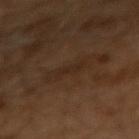{"biopsy_status": "not biopsied; imaged during a skin examination", "lesion_size": {"long_diameter_mm_approx": 2.5}, "image": {"source": "total-body photography crop", "field_of_view_mm": 15}, "automated_metrics": {"eccentricity": 0.9, "shape_asymmetry": 0.45, "cielab_L": 24, "cielab_a": 15, "cielab_b": 24, "vs_skin_darker_L": 4.0, "vs_skin_contrast_norm": 5.0, "border_irregularity_0_10": 5.0, "color_variation_0_10": 0.0, "peripheral_color_asymmetry": 0.0}, "patient": {"sex": "male", "age_approx": 65}}